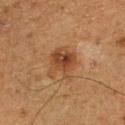Imaged during a routine full-body skin examination; the lesion was not biopsied and no histopathology is available. The tile uses cross-polarized illumination. A male subject in their mid-70s. About 4.5 mm across. On the right lower leg. Cropped from a whole-body photographic skin survey; the tile spans about 15 mm. An algorithmic analysis of the crop reported an area of roughly 11 mm² and an outline eccentricity of about 0.5 (0 = round, 1 = elongated). The software also gave about 8 CIELAB-L* units darker than the surrounding skin and a normalized lesion–skin contrast near 7.5. And it measured a lesion-detection confidence of about 100/100.